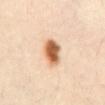No biopsy was performed on this lesion — it was imaged during a full skin examination and was not determined to be concerning. A male patient, in their mid-40s. A 15 mm close-up extracted from a 3D total-body photography capture. The lesion is on the abdomen. Automated image analysis of the tile measured border irregularity of about 1.5 on a 0–10 scale and peripheral color asymmetry of about 1.5. The software also gave lesion-presence confidence of about 100/100. Longest diameter approximately 3.5 mm. Captured under cross-polarized illumination.